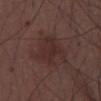The lesion was photographed on a routine skin check and not biopsied; there is no pathology result. Longest diameter approximately 4.5 mm. Captured under white-light illumination. A region of skin cropped from a whole-body photographic capture, roughly 15 mm wide. Located on the chest. The subject is a male roughly 30 years of age. An algorithmic analysis of the crop reported an average lesion color of about L≈28 a*≈18 b*≈18 (CIELAB), about 5 CIELAB-L* units darker than the surrounding skin, and a normalized border contrast of about 6. The software also gave a border-irregularity index near 5.5/10, a within-lesion color-variation index near 2/10, and radial color variation of about 1. The analysis additionally found a classifier nevus-likeness of about 0/100 and lesion-presence confidence of about 100/100.